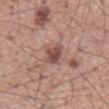Assessment:
Captured during whole-body skin photography for melanoma surveillance; the lesion was not biopsied.
Background:
The lesion's longest dimension is about 3 mm. A male patient, aged around 55. A roughly 15 mm field-of-view crop from a total-body skin photograph. This is a white-light tile. From the mid back.